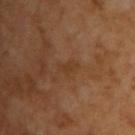Case summary:
• patient — male, aged approximately 65
• acquisition — ~15 mm tile from a whole-body skin photo
• illumination — cross-polarized